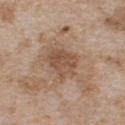The lesion was tiled from a total-body skin photograph and was not biopsied. Longest diameter approximately 3.5 mm. This is a white-light tile. A male patient, in their mid-60s. The lesion is on the chest. An algorithmic analysis of the crop reported a border-irregularity index near 2.5/10, a within-lesion color-variation index near 3.5/10, and peripheral color asymmetry of about 1. It also reported a nevus-likeness score of about 0/100 and a detector confidence of about 100 out of 100 that the crop contains a lesion. Cropped from a total-body skin-imaging series; the visible field is about 15 mm.